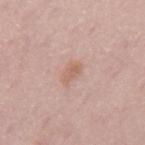Q: Lesion location?
A: the mid back
Q: What kind of image is this?
A: 15 mm crop, total-body photography
Q: Patient demographics?
A: male, in their mid- to late 50s
Q: Illumination type?
A: white-light illumination
Q: What is the lesion's diameter?
A: ≈2.5 mm